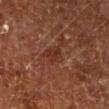workup = imaged on a skin check; not biopsied
location = the leg
subject = male, roughly 65 years of age
image = ~15 mm crop, total-body skin-cancer survey